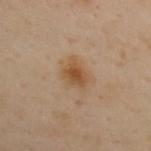Image and clinical context:
The lesion is located on the upper back. A female subject approximately 60 years of age. A 15 mm crop from a total-body photograph taken for skin-cancer surveillance. The lesion-visualizer software estimated a lesion color around L≈43 a*≈16 b*≈31 in CIELAB and about 8 CIELAB-L* units darker than the surrounding skin. The software also gave a border-irregularity index near 2.5/10 and a color-variation rating of about 3/10.